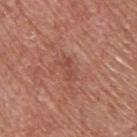  lesion_size:
    long_diameter_mm_approx: 3.0
  site: upper back
  image:
    source: total-body photography crop
    field_of_view_mm: 15
  patient:
    sex: male
    age_approx: 65